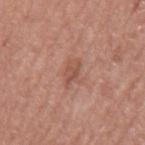The lesion was tiled from a total-body skin photograph and was not biopsied. The lesion is on the back. Longest diameter approximately 3 mm. A male patient in their mid- to late 70s. Automated tile analysis of the lesion measured an average lesion color of about L≈52 a*≈23 b*≈28 (CIELAB), about 8 CIELAB-L* units darker than the surrounding skin, and a normalized lesion–skin contrast near 6. The analysis additionally found a border-irregularity index near 3/10, a within-lesion color-variation index near 2.5/10, and a peripheral color-asymmetry measure near 0.5. The software also gave a nevus-likeness score of about 0/100 and lesion-presence confidence of about 100/100. Imaged with white-light lighting. A lesion tile, about 15 mm wide, cut from a 3D total-body photograph.